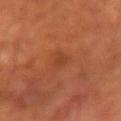notes = no biopsy performed (imaged during a skin exam) | patient = male, aged around 60 | image = total-body-photography crop, ~15 mm field of view | lesion size = about 2.5 mm | anatomic site = the right forearm | automated metrics = a symmetry-axis asymmetry near 0.3; a border-irregularity rating of about 3/10, internal color variation of about 0.5 on a 0–10 scale, and a peripheral color-asymmetry measure near 0; a classifier nevus-likeness of about 0/100 and a lesion-detection confidence of about 100/100 | lighting = cross-polarized illumination.